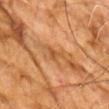Assessment:
No biopsy was performed on this lesion — it was imaged during a full skin examination and was not determined to be concerning.
Image and clinical context:
A roughly 15 mm field-of-view crop from a total-body skin photograph. The total-body-photography lesion software estimated a mean CIELAB color near L≈53 a*≈24 b*≈41 and a lesion–skin lightness drop of about 8. It also reported a classifier nevus-likeness of about 0/100 and a lesion-detection confidence of about 60/100. Imaged with cross-polarized lighting. Located on the chest. A male subject approximately 70 years of age.